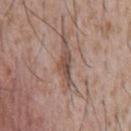No biopsy was performed on this lesion — it was imaged during a full skin examination and was not determined to be concerning.
From the chest.
Imaged with white-light lighting.
Approximately 3.5 mm at its widest.
The lesion-visualizer software estimated a lesion area of about 3.5 mm², an outline eccentricity of about 0.9 (0 = round, 1 = elongated), and a symmetry-axis asymmetry near 0.6. The software also gave a mean CIELAB color near L≈47 a*≈17 b*≈24. It also reported a nevus-likeness score of about 0/100 and lesion-presence confidence of about 90/100.
A male patient, in their mid-40s.
A lesion tile, about 15 mm wide, cut from a 3D total-body photograph.